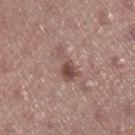Notes:
– diameter · about 4.5 mm
– lighting · white-light illumination
– location · the right lower leg
– patient · female, in their 20s
– image source · total-body-photography crop, ~15 mm field of view